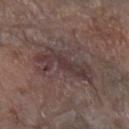{
  "biopsy_status": "not biopsied; imaged during a skin examination",
  "patient": {
    "sex": "female",
    "age_approx": "85+"
  },
  "site": "arm",
  "image": {
    "source": "total-body photography crop",
    "field_of_view_mm": 15
  }
}